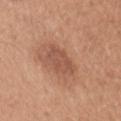Impression: Recorded during total-body skin imaging; not selected for excision or biopsy. Background: The lesion-visualizer software estimated a lesion area of about 7.5 mm², an outline eccentricity of about 0.6 (0 = round, 1 = elongated), and two-axis asymmetry of about 0.25. It also reported a mean CIELAB color near L≈52 a*≈23 b*≈30 and about 8 CIELAB-L* units darker than the surrounding skin. On the arm. The tile uses white-light illumination. A lesion tile, about 15 mm wide, cut from a 3D total-body photograph. The recorded lesion diameter is about 3.5 mm. A female patient, aged approximately 30.